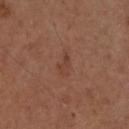| key | value |
|---|---|
| workup | total-body-photography surveillance lesion; no biopsy |
| image-analysis metrics | a border-irregularity rating of about 4.5/10, internal color variation of about 0.5 on a 0–10 scale, and peripheral color asymmetry of about 0 |
| lesion size | ≈3.5 mm |
| tile lighting | cross-polarized |
| imaging modality | total-body-photography crop, ~15 mm field of view |
| anatomic site | the upper back |
| patient | male, aged 43–47 |